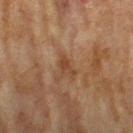This lesion was catalogued during total-body skin photography and was not selected for biopsy. Captured under cross-polarized illumination. From the left forearm. A female subject, approximately 80 years of age. Approximately 2.5 mm at its widest. A roughly 15 mm field-of-view crop from a total-body skin photograph.